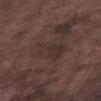notes = imaged on a skin check; not biopsied
image source = ~15 mm crop, total-body skin-cancer survey
illumination = white-light
TBP lesion metrics = a classifier nevus-likeness of about 0/100 and lesion-presence confidence of about 65/100
subject = male, aged approximately 75
location = the right thigh